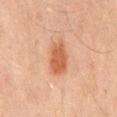Impression:
Captured during whole-body skin photography for melanoma surveillance; the lesion was not biopsied.
Clinical summary:
An algorithmic analysis of the crop reported a lesion–skin lightness drop of about 10 and a normalized border contrast of about 8. The lesion's longest dimension is about 4 mm. A male subject, aged 43–47. On the mid back. A 15 mm crop from a total-body photograph taken for skin-cancer surveillance. This is a cross-polarized tile.